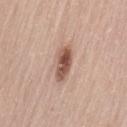| key | value |
|---|---|
| biopsy status | imaged on a skin check; not biopsied |
| automated lesion analysis | a classifier nevus-likeness of about 95/100 and a detector confidence of about 100 out of 100 that the crop contains a lesion |
| location | the back |
| imaging modality | ~15 mm tile from a whole-body skin photo |
| lesion size | about 4.5 mm |
| illumination | white-light illumination |
| patient | female, aged approximately 65 |